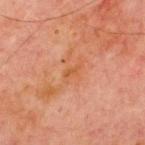The lesion was tiled from a total-body skin photograph and was not biopsied. Longest diameter approximately 2.5 mm. This is a cross-polarized tile. This image is a 15 mm lesion crop taken from a total-body photograph. The lesion-visualizer software estimated a footprint of about 2.5 mm² and two-axis asymmetry of about 0.45. The analysis additionally found a border-irregularity rating of about 4.5/10 and radial color variation of about 0. And it measured a classifier nevus-likeness of about 0/100 and lesion-presence confidence of about 100/100. Located on the chest. The subject is a male roughly 70 years of age.